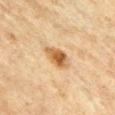Impression: Imaged during a routine full-body skin examination; the lesion was not biopsied and no histopathology is available.